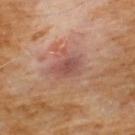Imaged during a routine full-body skin examination; the lesion was not biopsied and no histopathology is available. Imaged with cross-polarized lighting. A male subject approximately 60 years of age. The lesion's longest dimension is about 3.5 mm. The lesion is located on the chest. A region of skin cropped from a whole-body photographic capture, roughly 15 mm wide.